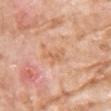Clinical impression:
This lesion was catalogued during total-body skin photography and was not selected for biopsy.
Image and clinical context:
On the chest. Captured under white-light illumination. This image is a 15 mm lesion crop taken from a total-body photograph. The subject is a female aged 73 to 77. Longest diameter approximately 3.5 mm.